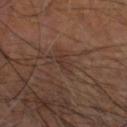The lesion was photographed on a routine skin check and not biopsied; there is no pathology result.
Measured at roughly 2.5 mm in maximum diameter.
A male patient aged around 65.
Automated tile analysis of the lesion measured an area of roughly 3 mm², an outline eccentricity of about 0.85 (0 = round, 1 = elongated), and two-axis asymmetry of about 0.35. And it measured a within-lesion color-variation index near 1/10 and radial color variation of about 0.5. The software also gave a detector confidence of about 65 out of 100 that the crop contains a lesion.
A 15 mm close-up extracted from a 3D total-body photography capture.
This is a cross-polarized tile.
On the head or neck.